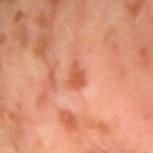notes: catalogued during a skin exam; not biopsied | subject: male, aged around 60 | lesion diameter: ~2.5 mm (longest diameter) | imaging modality: ~15 mm crop, total-body skin-cancer survey | automated metrics: an area of roughly 3.5 mm², an outline eccentricity of about 0.75 (0 = round, 1 = elongated), and a symmetry-axis asymmetry near 0.35; a classifier nevus-likeness of about 5/100 and a detector confidence of about 100 out of 100 that the crop contains a lesion | site: the right upper arm | illumination: cross-polarized illumination.